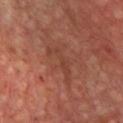This lesion was catalogued during total-body skin photography and was not selected for biopsy. About 5.5 mm across. From the chest. Cropped from a total-body skin-imaging series; the visible field is about 15 mm. The tile uses cross-polarized illumination. A patient roughly 65 years of age. The total-body-photography lesion software estimated a lesion area of about 6 mm², an outline eccentricity of about 0.95 (0 = round, 1 = elongated), and two-axis asymmetry of about 0.6.